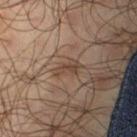TBP lesion metrics: a footprint of about 3.5 mm² and a shape-asymmetry score of about 0.4 (0 = symmetric); an average lesion color of about L≈40 a*≈15 b*≈25 (CIELAB), roughly 7 lightness units darker than nearby skin, and a normalized lesion–skin contrast near 6.5; a border-irregularity rating of about 4.5/10, internal color variation of about 2.5 on a 0–10 scale, and radial color variation of about 1; a classifier nevus-likeness of about 5/100 | acquisition: total-body-photography crop, ~15 mm field of view | patient: male, aged around 65 | diameter: about 3 mm | site: the leg | tile lighting: cross-polarized illumination.